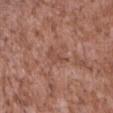No biopsy was performed on this lesion — it was imaged during a full skin examination and was not determined to be concerning.
A male subject in their mid- to late 40s.
Located on the abdomen.
Captured under white-light illumination.
An algorithmic analysis of the crop reported a mean CIELAB color near L≈48 a*≈22 b*≈27, a lesion–skin lightness drop of about 7, and a normalized lesion–skin contrast near 5. The software also gave border irregularity of about 4.5 on a 0–10 scale and peripheral color asymmetry of about 0.
A lesion tile, about 15 mm wide, cut from a 3D total-body photograph.
The recorded lesion diameter is about 3 mm.